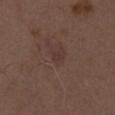Assessment: This lesion was catalogued during total-body skin photography and was not selected for biopsy. Image and clinical context: The lesion is located on the right thigh. The lesion-visualizer software estimated an area of roughly 1 mm², an outline eccentricity of about 0.6 (0 = round, 1 = elongated), and a symmetry-axis asymmetry near 0.35. A roughly 15 mm field-of-view crop from a total-body skin photograph. About 1 mm across. A female patient aged approximately 40.